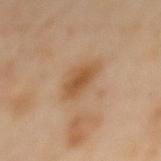Q: Was this lesion biopsied?
A: no biopsy performed (imaged during a skin exam)